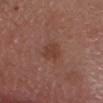{
  "biopsy_status": "not biopsied; imaged during a skin examination",
  "image": {
    "source": "total-body photography crop",
    "field_of_view_mm": 15
  },
  "site": "head or neck",
  "lesion_size": {
    "long_diameter_mm_approx": 3.0
  },
  "patient": {
    "sex": "male",
    "age_approx": 55
  },
  "lighting": "white-light"
}